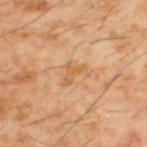Impression:
No biopsy was performed on this lesion — it was imaged during a full skin examination and was not determined to be concerning.
Context:
The subject is a male aged approximately 60. Approximately 3 mm at its widest. A lesion tile, about 15 mm wide, cut from a 3D total-body photograph. The lesion is located on the upper back.